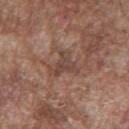No biopsy was performed on this lesion — it was imaged during a full skin examination and was not determined to be concerning. The tile uses white-light illumination. Approximately 3.5 mm at its widest. A lesion tile, about 15 mm wide, cut from a 3D total-body photograph. The lesion is on the chest. A male patient approximately 75 years of age.